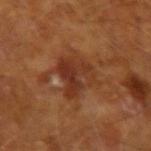The lesion was tiled from a total-body skin photograph and was not biopsied.
The subject is a male aged around 65.
A roughly 15 mm field-of-view crop from a total-body skin photograph.
On the left upper arm.